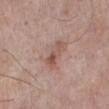Captured during whole-body skin photography for melanoma surveillance; the lesion was not biopsied.
A female patient, aged 63–67.
The total-body-photography lesion software estimated a lesion color around L≈53 a*≈20 b*≈25 in CIELAB, about 9 CIELAB-L* units darker than the surrounding skin, and a normalized border contrast of about 6.5. The analysis additionally found a border-irregularity rating of about 5/10, a within-lesion color-variation index near 2/10, and a peripheral color-asymmetry measure near 0.5.
Imaged with white-light lighting.
On the leg.
Approximately 3.5 mm at its widest.
A roughly 15 mm field-of-view crop from a total-body skin photograph.